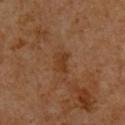Imaged during a routine full-body skin examination; the lesion was not biopsied and no histopathology is available. Located on the upper back. A lesion tile, about 15 mm wide, cut from a 3D total-body photograph. The lesion's longest dimension is about 2.5 mm. Automated tile analysis of the lesion measured a lesion area of about 4 mm² and a shape eccentricity near 0.75. The analysis additionally found border irregularity of about 2.5 on a 0–10 scale, a within-lesion color-variation index near 1.5/10, and radial color variation of about 0.5. And it measured a classifier nevus-likeness of about 0/100 and a detector confidence of about 100 out of 100 that the crop contains a lesion. A male patient aged approximately 60. Captured under cross-polarized illumination.